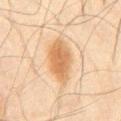{"biopsy_status": "not biopsied; imaged during a skin examination", "image": {"source": "total-body photography crop", "field_of_view_mm": 15}, "patient": {"sex": "male", "age_approx": 45}, "automated_metrics": {"area_mm2_approx": 14.0, "eccentricity": 0.85, "shape_asymmetry": 0.15, "cielab_L": 55, "cielab_a": 17, "cielab_b": 34, "vs_skin_darker_L": 10.0, "vs_skin_contrast_norm": 8.0, "color_variation_0_10": 3.5, "peripheral_color_asymmetry": 1.0, "nevus_likeness_0_100": 100}, "site": "abdomen"}